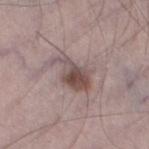Case summary:
– image: total-body-photography crop, ~15 mm field of view
– lighting: white-light
– site: the left thigh
– patient: male, approximately 70 years of age